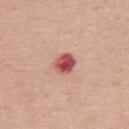Impression:
Recorded during total-body skin imaging; not selected for excision or biopsy.
Acquisition and patient details:
The total-body-photography lesion software estimated a border-irregularity rating of about 1.5/10, a within-lesion color-variation index near 6.5/10, and radial color variation of about 2. A male subject, in their mid-40s. The lesion is located on the back. Imaged with white-light lighting. Cropped from a whole-body photographic skin survey; the tile spans about 15 mm. Longest diameter approximately 3 mm.